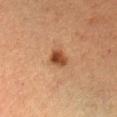Clinical impression:
Captured during whole-body skin photography for melanoma surveillance; the lesion was not biopsied.
Context:
From the left forearm. A 15 mm crop from a total-body photograph taken for skin-cancer surveillance. The subject is a female approximately 60 years of age.